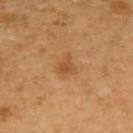Imaged during a routine full-body skin examination; the lesion was not biopsied and no histopathology is available. Measured at roughly 2.5 mm in maximum diameter. A female subject, aged around 55. Imaged with cross-polarized lighting. Automated tile analysis of the lesion measured a footprint of about 4 mm², an outline eccentricity of about 0.5 (0 = round, 1 = elongated), and two-axis asymmetry of about 0.35. It also reported an automated nevus-likeness rating near 20 out of 100 and lesion-presence confidence of about 100/100. This image is a 15 mm lesion crop taken from a total-body photograph. From the upper back.